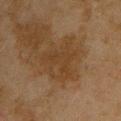The lesion was photographed on a routine skin check and not biopsied; there is no pathology result. A lesion tile, about 15 mm wide, cut from a 3D total-body photograph. Captured under cross-polarized illumination. Longest diameter approximately 5 mm. The lesion is located on the upper back. The patient is a male aged approximately 45.